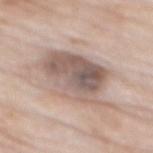follow-up = imaged on a skin check; not biopsied | image = ~15 mm crop, total-body skin-cancer survey | subject = male, aged approximately 85 or older | lesion size = ≈8 mm | location = the mid back | TBP lesion metrics = a classifier nevus-likeness of about 10/100 and a detector confidence of about 95 out of 100 that the crop contains a lesion | tile lighting = white-light.